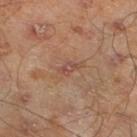<case>
<biopsy_status>not biopsied; imaged during a skin examination</biopsy_status>
<automated_metrics>
  <cielab_L>47</cielab_L>
  <cielab_a>21</cielab_a>
  <cielab_b>26</cielab_b>
  <vs_skin_darker_L>7.0</vs_skin_darker_L>
  <vs_skin_contrast_norm>6.0</vs_skin_contrast_norm>
  <border_irregularity_0_10>4.0</border_irregularity_0_10>
  <color_variation_0_10>0.0</color_variation_0_10>
  <peripheral_color_asymmetry>0.0</peripheral_color_asymmetry>
  <nevus_likeness_0_100>0</nevus_likeness_0_100>
</automated_metrics>
<site>left lower leg</site>
<lighting>cross-polarized</lighting>
<lesion_size>
  <long_diameter_mm_approx>2.5</long_diameter_mm_approx>
</lesion_size>
<patient>
  <sex>male</sex>
  <age_approx>45</age_approx>
</patient>
<image>
  <source>total-body photography crop</source>
  <field_of_view_mm>15</field_of_view_mm>
</image>
</case>